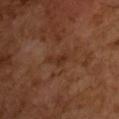biopsy status: no biopsy performed (imaged during a skin exam) | image source: ~15 mm tile from a whole-body skin photo | TBP lesion metrics: a lesion color around L≈30 a*≈21 b*≈28 in CIELAB, roughly 6 lightness units darker than nearby skin, and a lesion-to-skin contrast of about 6 (normalized; higher = more distinct) | lesion size: about 2.5 mm | subject: male, in their mid-60s.